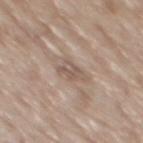  biopsy_status: not biopsied; imaged during a skin examination
  image:
    source: total-body photography crop
    field_of_view_mm: 15
  lesion_size:
    long_diameter_mm_approx: 3.0
  patient:
    sex: male
    age_approx: 70
  lighting: white-light
  site: mid back
  automated_metrics:
    border_irregularity_0_10: 4.5
    color_variation_0_10: 3.5
    peripheral_color_asymmetry: 1.5